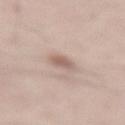This lesion was catalogued during total-body skin photography and was not selected for biopsy. On the lower back. A male patient, aged 48–52. A region of skin cropped from a whole-body photographic capture, roughly 15 mm wide. Automated image analysis of the tile measured an outline eccentricity of about 0.75 (0 = round, 1 = elongated) and a symmetry-axis asymmetry near 0.2. And it measured an average lesion color of about L≈60 a*≈17 b*≈24 (CIELAB) and a lesion–skin lightness drop of about 10. Captured under white-light illumination.